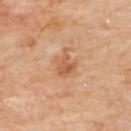The subject is a female aged approximately 60. Imaged with cross-polarized lighting. Longest diameter approximately 3.5 mm. This image is a 15 mm lesion crop taken from a total-body photograph. Automated image analysis of the tile measured a border-irregularity index near 3.5/10, internal color variation of about 4 on a 0–10 scale, and a peripheral color-asymmetry measure near 1.5. The software also gave a classifier nevus-likeness of about 15/100 and a detector confidence of about 100 out of 100 that the crop contains a lesion. On the upper back.